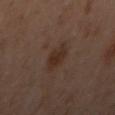The lesion was photographed on a routine skin check and not biopsied; there is no pathology result.
The lesion is on the left arm.
About 3.5 mm across.
An algorithmic analysis of the crop reported a footprint of about 7 mm², a shape eccentricity near 0.7, and two-axis asymmetry of about 0.2. The software also gave a lesion color around L≈30 a*≈16 b*≈24 in CIELAB, about 6 CIELAB-L* units darker than the surrounding skin, and a normalized lesion–skin contrast near 7. The analysis additionally found a nevus-likeness score of about 55/100 and lesion-presence confidence of about 100/100.
A female subject in their 40s.
A 15 mm crop from a total-body photograph taken for skin-cancer surveillance.